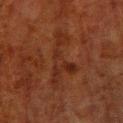automated lesion analysis: a shape eccentricity near 0.95 and a symmetry-axis asymmetry near 0.55; border irregularity of about 9 on a 0–10 scale and a color-variation rating of about 3/10; a nevus-likeness score of about 0/100 and a detector confidence of about 55 out of 100 that the crop contains a lesion | lighting: cross-polarized | body site: the right lower leg | patient: male, approximately 80 years of age | lesion diameter: ≈8 mm | acquisition: 15 mm crop, total-body photography.